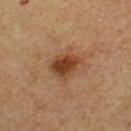Recorded during total-body skin imaging; not selected for excision or biopsy.
The lesion's longest dimension is about 3.5 mm.
A roughly 15 mm field-of-view crop from a total-body skin photograph.
On the front of the torso.
Imaged with cross-polarized lighting.
A male patient, aged approximately 65.